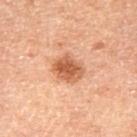notes — catalogued during a skin exam; not biopsied | lesion size — about 3.5 mm | subject — male, about 70 years old | TBP lesion metrics — a mean CIELAB color near L≈45 a*≈20 b*≈29 and about 10 CIELAB-L* units darker than the surrounding skin; a border-irregularity index near 2/10, internal color variation of about 3.5 on a 0–10 scale, and radial color variation of about 1 | illumination — cross-polarized | site — the right thigh | acquisition — ~15 mm crop, total-body skin-cancer survey.